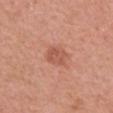The lesion was photographed on a routine skin check and not biopsied; there is no pathology result. The subject is a female about 40 years old. Approximately 3 mm at its widest. The lesion is located on the left forearm. A region of skin cropped from a whole-body photographic capture, roughly 15 mm wide. The tile uses white-light illumination. The lesion-visualizer software estimated two-axis asymmetry of about 0.2. The analysis additionally found an automated nevus-likeness rating near 35 out of 100.